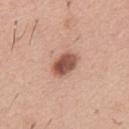Q: Was this lesion biopsied?
A: no biopsy performed (imaged during a skin exam)
Q: What is the anatomic site?
A: the mid back
Q: How was the tile lit?
A: white-light illumination
Q: What is the lesion's diameter?
A: ~3.5 mm (longest diameter)
Q: What kind of image is this?
A: total-body-photography crop, ~15 mm field of view
Q: Patient demographics?
A: male, about 40 years old